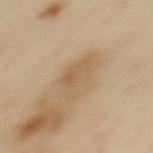| key | value |
|---|---|
| follow-up | no biopsy performed (imaged during a skin exam) |
| diameter | ~5 mm (longest diameter) |
| location | the upper back |
| lighting | cross-polarized |
| image source | ~15 mm tile from a whole-body skin photo |
| subject | female, aged 38 to 42 |